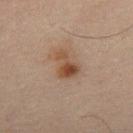{
  "biopsy_status": "not biopsied; imaged during a skin examination",
  "lesion_size": {
    "long_diameter_mm_approx": 3.5
  },
  "site": "right thigh",
  "image": {
    "source": "total-body photography crop",
    "field_of_view_mm": 15
  },
  "lighting": "cross-polarized",
  "patient": {
    "sex": "male",
    "age_approx": 65
  }
}